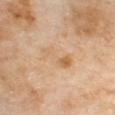Image and clinical context:
A close-up tile cropped from a whole-body skin photograph, about 15 mm across. The recorded lesion diameter is about 4.5 mm. Imaged with cross-polarized lighting. A male patient roughly 70 years of age. The lesion is on the front of the torso.